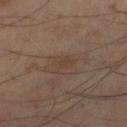Q: Was this lesion biopsied?
A: imaged on a skin check; not biopsied
Q: Where on the body is the lesion?
A: the right lower leg
Q: What is the imaging modality?
A: ~15 mm crop, total-body skin-cancer survey
Q: How was the tile lit?
A: cross-polarized illumination
Q: Who is the patient?
A: male, roughly 55 years of age